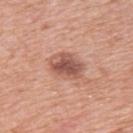follow-up: no biopsy performed (imaged during a skin exam) | lighting: white-light illumination | site: the upper back | image-analysis metrics: a border-irregularity rating of about 2/10 and a peripheral color-asymmetry measure near 1.5; an automated nevus-likeness rating near 50 out of 100 | image source: 15 mm crop, total-body photography | patient: female, aged around 40.